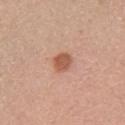- biopsy status — catalogued during a skin exam; not biopsied
- lighting — white-light illumination
- location — the left upper arm
- subject — female, aged 43–47
- acquisition — ~15 mm tile from a whole-body skin photo
- diameter — ~2.5 mm (longest diameter)